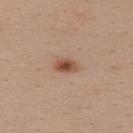Impression: This lesion was catalogued during total-body skin photography and was not selected for biopsy. Background: The lesion is located on the upper back. A female subject aged approximately 25. Approximately 3 mm at its widest. The lesion-visualizer software estimated a border-irregularity index near 2/10, internal color variation of about 4.5 on a 0–10 scale, and radial color variation of about 1.5. The analysis additionally found an automated nevus-likeness rating near 100 out of 100 and a lesion-detection confidence of about 100/100. A roughly 15 mm field-of-view crop from a total-body skin photograph. This is a white-light tile.